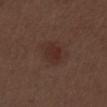Impression:
Part of a total-body skin-imaging series; this lesion was reviewed on a skin check and was not flagged for biopsy.
Clinical summary:
The recorded lesion diameter is about 3 mm. The tile uses white-light illumination. A lesion tile, about 15 mm wide, cut from a 3D total-body photograph. Automated tile analysis of the lesion measured a footprint of about 5 mm², a shape eccentricity near 0.7, and a symmetry-axis asymmetry near 0.25. The software also gave a lesion color around L≈28 a*≈19 b*≈22 in CIELAB and a lesion-to-skin contrast of about 6.5 (normalized; higher = more distinct). And it measured a nevus-likeness score of about 35/100 and a detector confidence of about 100 out of 100 that the crop contains a lesion. Located on the left thigh. A male patient, aged 68 to 72.